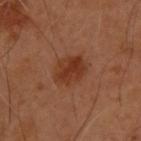Notes:
- follow-up: total-body-photography surveillance lesion; no biopsy
- imaging modality: 15 mm crop, total-body photography
- site: the upper back
- subject: male, aged 53 to 57
- automated metrics: an area of roughly 9 mm², an eccentricity of roughly 0.65, and a shape-asymmetry score of about 0.2 (0 = symmetric); a mean CIELAB color near L≈34 a*≈24 b*≈30, about 8 CIELAB-L* units darker than the surrounding skin, and a normalized lesion–skin contrast near 8; a nevus-likeness score of about 90/100 and lesion-presence confidence of about 100/100
- diameter: ~4 mm (longest diameter)
- tile lighting: cross-polarized illumination